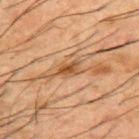Imaged during a routine full-body skin examination; the lesion was not biopsied and no histopathology is available. Located on the back. A 15 mm close-up tile from a total-body photography series done for melanoma screening. Longest diameter approximately 2.5 mm. A male patient aged 48 to 52.